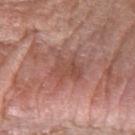Clinical impression: No biopsy was performed on this lesion — it was imaged during a full skin examination and was not determined to be concerning. Context: A male subject, about 75 years old. A region of skin cropped from a whole-body photographic capture, roughly 15 mm wide. The lesion is located on the right forearm. An algorithmic analysis of the crop reported a footprint of about 10 mm², an outline eccentricity of about 0.4 (0 = round, 1 = elongated), and two-axis asymmetry of about 0.3. And it measured a mean CIELAB color near L≈50 a*≈24 b*≈26, a lesion–skin lightness drop of about 7, and a normalized border contrast of about 5.5. The software also gave border irregularity of about 5 on a 0–10 scale, internal color variation of about 3.5 on a 0–10 scale, and a peripheral color-asymmetry measure near 1.5.